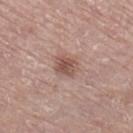Captured during whole-body skin photography for melanoma surveillance; the lesion was not biopsied. A 15 mm close-up extracted from a 3D total-body photography capture. From the right lower leg. The recorded lesion diameter is about 2.5 mm. A female subject, aged around 75.